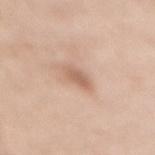Part of a total-body skin-imaging series; this lesion was reviewed on a skin check and was not flagged for biopsy.
This image is a 15 mm lesion crop taken from a total-body photograph.
This is a white-light tile.
Measured at roughly 3 mm in maximum diameter.
The patient is a female aged around 35.
On the back.